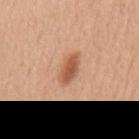Clinical impression: Captured during whole-body skin photography for melanoma surveillance; the lesion was not biopsied. Clinical summary: From the mid back. This is a white-light tile. Longest diameter approximately 4 mm. Cropped from a total-body skin-imaging series; the visible field is about 15 mm. The patient is a male approximately 50 years of age.